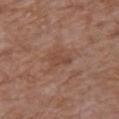Clinical impression:
The lesion was photographed on a routine skin check and not biopsied; there is no pathology result.
Clinical summary:
Cropped from a whole-body photographic skin survey; the tile spans about 15 mm. A male subject about 60 years old. The lesion is located on the upper back.